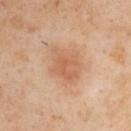- follow-up · total-body-photography surveillance lesion; no biopsy
- illumination · cross-polarized illumination
- location · the chest
- diameter · ≈4.5 mm
- imaging modality · 15 mm crop, total-body photography
- automated lesion analysis · a border-irregularity rating of about 2.5/10, a within-lesion color-variation index near 2.5/10, and peripheral color asymmetry of about 1
- patient · male, roughly 55 years of age